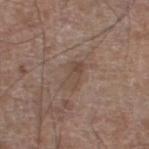biopsy status — imaged on a skin check; not biopsied | illumination — white-light illumination | imaging modality — 15 mm crop, total-body photography | anatomic site — the leg | subject — male, aged 58 to 62.